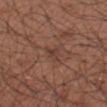<tbp_lesion>
  <biopsy_status>not biopsied; imaged during a skin examination</biopsy_status>
  <image>
    <source>total-body photography crop</source>
    <field_of_view_mm>15</field_of_view_mm>
  </image>
  <site>right forearm</site>
  <patient>
    <sex>male</sex>
    <age_approx>55</age_approx>
  </patient>
</tbp_lesion>